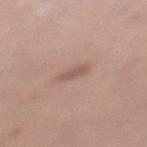<tbp_lesion>
<biopsy_status>not biopsied; imaged during a skin examination</biopsy_status>
<lighting>white-light</lighting>
<image>
  <source>total-body photography crop</source>
  <field_of_view_mm>15</field_of_view_mm>
</image>
<automated_metrics>
  <area_mm2_approx>3.0</area_mm2_approx>
  <eccentricity>0.85</eccentricity>
  <shape_asymmetry>0.3</shape_asymmetry>
  <cielab_L>55</cielab_L>
  <cielab_a>19</cielab_a>
  <cielab_b>24</cielab_b>
  <vs_skin_darker_L>8.0</vs_skin_darker_L>
  <vs_skin_contrast_norm>6.0</vs_skin_contrast_norm>
  <border_irregularity_0_10>3.0</border_irregularity_0_10>
  <color_variation_0_10>1.0</color_variation_0_10>
  <nevus_likeness_0_100>0</nevus_likeness_0_100>
</automated_metrics>
<site>left lower leg</site>
<patient>
  <sex>female</sex>
  <age_approx>60</age_approx>
</patient>
</tbp_lesion>